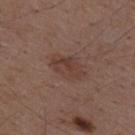This lesion was catalogued during total-body skin photography and was not selected for biopsy.
About 4 mm across.
The lesion is on the mid back.
The lesion-visualizer software estimated a lesion area of about 9 mm², an outline eccentricity of about 0.8 (0 = round, 1 = elongated), and a symmetry-axis asymmetry near 0.2. It also reported border irregularity of about 2 on a 0–10 scale, a within-lesion color-variation index near 3/10, and radial color variation of about 1.5. The analysis additionally found a classifier nevus-likeness of about 35/100.
Cropped from a whole-body photographic skin survey; the tile spans about 15 mm.
A male subject aged approximately 50.
Imaged with white-light lighting.